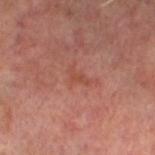Clinical impression: Captured during whole-body skin photography for melanoma surveillance; the lesion was not biopsied.